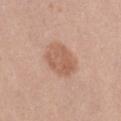<tbp_lesion>
  <biopsy_status>not biopsied; imaged during a skin examination</biopsy_status>
  <automated_metrics>
    <eccentricity>0.6</eccentricity>
    <shape_asymmetry>0.15</shape_asymmetry>
    <nevus_likeness_0_100>15</nevus_likeness_0_100>
    <lesion_detection_confidence_0_100>100</lesion_detection_confidence_0_100>
  </automated_metrics>
  <image>
    <source>total-body photography crop</source>
    <field_of_view_mm>15</field_of_view_mm>
  </image>
  <site>right thigh</site>
  <patient>
    <sex>female</sex>
    <age_approx>20</age_approx>
  </patient>
  <lesion_size>
    <long_diameter_mm_approx>4.0</long_diameter_mm_approx>
  </lesion_size>
</tbp_lesion>